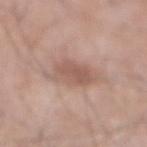automated metrics: an area of roughly 12 mm², an eccentricity of roughly 0.65, and two-axis asymmetry of about 0.25; a peripheral color-asymmetry measure near 1; a nevus-likeness score of about 25/100 and lesion-presence confidence of about 100/100
size: about 4.5 mm
anatomic site: the abdomen
patient: male, aged around 70
acquisition: 15 mm crop, total-body photography
tile lighting: white-light illumination